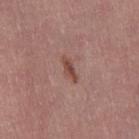{
  "biopsy_status": "not biopsied; imaged during a skin examination",
  "lighting": "white-light",
  "site": "leg",
  "lesion_size": {
    "long_diameter_mm_approx": 3.5
  },
  "patient": {
    "sex": "female",
    "age_approx": 50
  },
  "automated_metrics": {
    "color_variation_0_10": 1.5,
    "peripheral_color_asymmetry": 0.5,
    "nevus_likeness_0_100": 0,
    "lesion_detection_confidence_0_100": 100
  },
  "image": {
    "source": "total-body photography crop",
    "field_of_view_mm": 15
  }
}